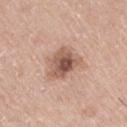Q: Is there a histopathology result?
A: imaged on a skin check; not biopsied
Q: What lighting was used for the tile?
A: white-light illumination
Q: Patient demographics?
A: male, approximately 75 years of age
Q: Lesion location?
A: the right thigh
Q: What is the imaging modality?
A: ~15 mm crop, total-body skin-cancer survey